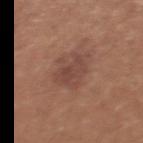Clinical impression:
The lesion was photographed on a routine skin check and not biopsied; there is no pathology result.
Background:
Located on the chest. A 15 mm close-up tile from a total-body photography series done for melanoma screening. The lesion's longest dimension is about 4 mm. A female patient, aged 33–37. Imaged with white-light lighting.